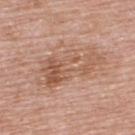Q: Is there a histopathology result?
A: total-body-photography surveillance lesion; no biopsy
Q: What is the anatomic site?
A: the upper back
Q: Automated lesion metrics?
A: a lesion–skin lightness drop of about 8 and a normalized lesion–skin contrast near 6; border irregularity of about 5.5 on a 0–10 scale, a color-variation rating of about 7/10, and radial color variation of about 2.5; an automated nevus-likeness rating near 0 out of 100 and a detector confidence of about 100 out of 100 that the crop contains a lesion
Q: Who is the patient?
A: male, aged 68–72
Q: Lesion size?
A: ~8 mm (longest diameter)
Q: Illumination type?
A: white-light illumination
Q: What is the imaging modality?
A: ~15 mm crop, total-body skin-cancer survey